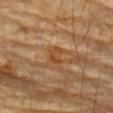workup = catalogued during a skin exam; not biopsied | subject = male, in their mid-80s or older | location = the left forearm | image = ~15 mm crop, total-body skin-cancer survey.